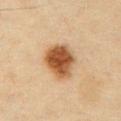This lesion was catalogued during total-body skin photography and was not selected for biopsy.
Longest diameter approximately 4.5 mm.
Cropped from a whole-body photographic skin survey; the tile spans about 15 mm.
The patient is a male in their 40s.
The tile uses cross-polarized illumination.
The lesion-visualizer software estimated a lesion color around L≈48 a*≈21 b*≈35 in CIELAB, a lesion–skin lightness drop of about 16, and a lesion-to-skin contrast of about 12 (normalized; higher = more distinct). It also reported a border-irregularity index near 1.5/10, internal color variation of about 5 on a 0–10 scale, and radial color variation of about 1.5.
Located on the chest.